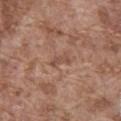No biopsy was performed on this lesion — it was imaged during a full skin examination and was not determined to be concerning. Measured at roughly 2.5 mm in maximum diameter. A 15 mm close-up extracted from a 3D total-body photography capture. Automated image analysis of the tile measured a footprint of about 2 mm² and a shape eccentricity near 0.95. And it measured an average lesion color of about L≈49 a*≈20 b*≈27 (CIELAB), about 8 CIELAB-L* units darker than the surrounding skin, and a lesion-to-skin contrast of about 6 (normalized; higher = more distinct). It also reported a border-irregularity rating of about 5/10, a within-lesion color-variation index near 0/10, and peripheral color asymmetry of about 0. The analysis additionally found a classifier nevus-likeness of about 0/100 and lesion-presence confidence of about 95/100. Located on the abdomen. The subject is a male about 75 years old.